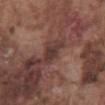* notes — catalogued during a skin exam; not biopsied
* lesion size — about 3.5 mm
* body site — the abdomen
* lighting — white-light
* automated lesion analysis — an area of roughly 5 mm², an outline eccentricity of about 0.8 (0 = round, 1 = elongated), and a symmetry-axis asymmetry near 0.35; a nevus-likeness score of about 0/100 and a detector confidence of about 70 out of 100 that the crop contains a lesion
* patient — male, aged approximately 75
* acquisition — 15 mm crop, total-body photography